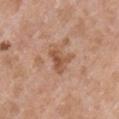| key | value |
|---|---|
| notes | catalogued during a skin exam; not biopsied |
| anatomic site | the front of the torso |
| acquisition | total-body-photography crop, ~15 mm field of view |
| patient | male, in their mid-30s |